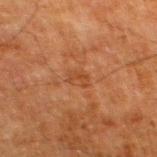Assessment:
No biopsy was performed on this lesion — it was imaged during a full skin examination and was not determined to be concerning.
Image and clinical context:
A male subject, approximately 80 years of age. Captured under cross-polarized illumination. The total-body-photography lesion software estimated a lesion-detection confidence of about 100/100. The lesion's longest dimension is about 2.5 mm. A roughly 15 mm field-of-view crop from a total-body skin photograph. On the left thigh.